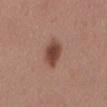* follow-up — catalogued during a skin exam; not biopsied
* patient — female, in their mid-50s
* tile lighting — white-light illumination
* location — the back
* image — ~15 mm crop, total-body skin-cancer survey